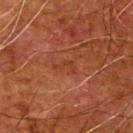workup — total-body-photography surveillance lesion; no biopsy | site — the left upper arm | imaging modality — ~15 mm tile from a whole-body skin photo | TBP lesion metrics — a lesion area of about 3 mm², an eccentricity of roughly 0.9, and a symmetry-axis asymmetry near 0.65; about 4 CIELAB-L* units darker than the surrounding skin and a normalized lesion–skin contrast near 4.5; border irregularity of about 7 on a 0–10 scale, a color-variation rating of about 0/10, and radial color variation of about 0; an automated nevus-likeness rating near 0 out of 100 | tile lighting — cross-polarized | subject — male, approximately 80 years of age | diameter — about 3 mm.